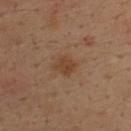Assessment: Recorded during total-body skin imaging; not selected for excision or biopsy. Clinical summary: The subject is a male in their mid- to late 30s. From the upper back. Measured at roughly 2.5 mm in maximum diameter. A lesion tile, about 15 mm wide, cut from a 3D total-body photograph.